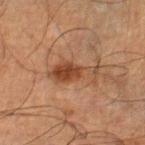Clinical impression:
The lesion was tiled from a total-body skin photograph and was not biopsied.
Acquisition and patient details:
The tile uses cross-polarized illumination. From the left lower leg. Measured at roughly 5.5 mm in maximum diameter. A region of skin cropped from a whole-body photographic capture, roughly 15 mm wide. A male subject, roughly 65 years of age.